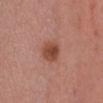| field | value |
|---|---|
| workup | total-body-photography surveillance lesion; no biopsy |
| diameter | ≈3 mm |
| subject | female, about 35 years old |
| image | ~15 mm crop, total-body skin-cancer survey |
| illumination | white-light illumination |
| site | the front of the torso |